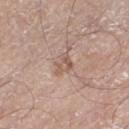tile lighting = white-light | location = the leg | patient = male, aged approximately 70 | image source = total-body-photography crop, ~15 mm field of view | lesion diameter = about 2.5 mm.